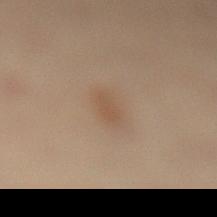tile lighting: cross-polarized; location: the left lower leg; acquisition: ~15 mm tile from a whole-body skin photo; diameter: ~2.5 mm (longest diameter); patient: female, about 40 years old.